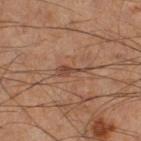biopsy status: no biopsy performed (imaged during a skin exam) | anatomic site: the left thigh | TBP lesion metrics: an outline eccentricity of about 0.95 (0 = round, 1 = elongated) and a shape-asymmetry score of about 0.55 (0 = symmetric); a border-irregularity rating of about 6.5/10, a color-variation rating of about 2.5/10, and radial color variation of about 1; a nevus-likeness score of about 0/100 and a lesion-detection confidence of about 95/100 | size: about 4 mm | subject: male, approximately 60 years of age | imaging modality: ~15 mm tile from a whole-body skin photo.